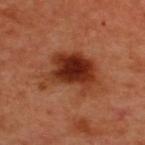This lesion was catalogued during total-body skin photography and was not selected for biopsy.
This image is a 15 mm lesion crop taken from a total-body photograph.
Located on the upper back.
A male subject, aged around 50.